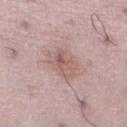notes: total-body-photography surveillance lesion; no biopsy | subject: male, in their 50s | automated lesion analysis: an area of roughly 11 mm², a shape eccentricity near 0.65, and a shape-asymmetry score of about 0.15 (0 = symmetric); a mean CIELAB color near L≈59 a*≈19 b*≈22, a lesion–skin lightness drop of about 8, and a lesion-to-skin contrast of about 6 (normalized; higher = more distinct) | image: ~15 mm crop, total-body skin-cancer survey | location: the right lower leg | tile lighting: white-light illumination.